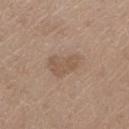Impression: No biopsy was performed on this lesion — it was imaged during a full skin examination and was not determined to be concerning. Clinical summary: The patient is a male aged 68–72. An algorithmic analysis of the crop reported an area of roughly 10 mm² and a symmetry-axis asymmetry near 0.3. It also reported an average lesion color of about L≈54 a*≈15 b*≈28 (CIELAB), about 7 CIELAB-L* units darker than the surrounding skin, and a normalized lesion–skin contrast near 5. And it measured a border-irregularity index near 3/10, internal color variation of about 2 on a 0–10 scale, and a peripheral color-asymmetry measure near 0.5. The software also gave an automated nevus-likeness rating near 0 out of 100 and a detector confidence of about 100 out of 100 that the crop contains a lesion. Approximately 4.5 mm at its widest. On the left thigh. Cropped from a whole-body photographic skin survey; the tile spans about 15 mm.